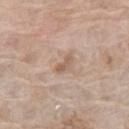Imaged during a routine full-body skin examination; the lesion was not biopsied and no histopathology is available.
The patient is a female approximately 75 years of age.
Longest diameter approximately 2.5 mm.
A close-up tile cropped from a whole-body skin photograph, about 15 mm across.
This is a white-light tile.
From the leg.